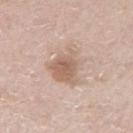{
  "patient": {
    "sex": "male",
    "age_approx": 75
  },
  "lighting": "white-light",
  "lesion_size": {
    "long_diameter_mm_approx": 5.0
  },
  "image": {
    "source": "total-body photography crop",
    "field_of_view_mm": 15
  },
  "automated_metrics": {
    "area_mm2_approx": 14.0,
    "shape_asymmetry": 0.35,
    "vs_skin_darker_L": 9.0,
    "vs_skin_contrast_norm": 6.5,
    "lesion_detection_confidence_0_100": 100
  },
  "site": "arm"
}